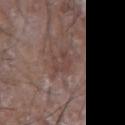Case summary:
* follow-up · total-body-photography surveillance lesion; no biopsy
* tile lighting · white-light
* patient · male, about 80 years old
* TBP lesion metrics · an outline eccentricity of about 0.85 (0 = round, 1 = elongated) and a symmetry-axis asymmetry near 0.35; an average lesion color of about L≈42 a*≈17 b*≈21 (CIELAB), roughly 6 lightness units darker than nearby skin, and a normalized lesion–skin contrast near 5; a classifier nevus-likeness of about 0/100 and a detector confidence of about 80 out of 100 that the crop contains a lesion
* acquisition · ~15 mm tile from a whole-body skin photo
* lesion size · ~3.5 mm (longest diameter)
* anatomic site · the left forearm